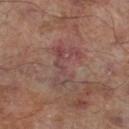follow-up: imaged on a skin check; not biopsied | subject: male, in their mid-60s | illumination: cross-polarized illumination | acquisition: total-body-photography crop, ~15 mm field of view | body site: the right lower leg.